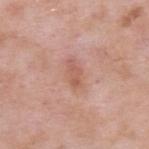Findings:
* workup: catalogued during a skin exam; not biopsied
* location: the back
* image-analysis metrics: a footprint of about 3 mm² and an eccentricity of roughly 0.9; a mean CIELAB color near L≈57 a*≈24 b*≈29, a lesion–skin lightness drop of about 8, and a normalized lesion–skin contrast near 6; a border-irregularity rating of about 3/10, a color-variation rating of about 0/10, and radial color variation of about 0
* image source: total-body-photography crop, ~15 mm field of view
* lighting: white-light illumination
* patient: male, roughly 55 years of age